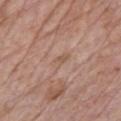Q: Is there a histopathology result?
A: catalogued during a skin exam; not biopsied
Q: Lesion size?
A: about 2.5 mm
Q: Automated lesion metrics?
A: a shape eccentricity near 0.9 and a shape-asymmetry score of about 0.3 (0 = symmetric); a border-irregularity index near 2.5/10, a within-lesion color-variation index near 1/10, and radial color variation of about 0; a classifier nevus-likeness of about 0/100 and lesion-presence confidence of about 95/100
Q: What is the anatomic site?
A: the chest
Q: What kind of image is this?
A: 15 mm crop, total-body photography
Q: Who is the patient?
A: female, roughly 70 years of age
Q: Illumination type?
A: white-light illumination